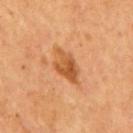<tbp_lesion>
  <patient>
    <sex>male</sex>
    <age_approx>55</age_approx>
  </patient>
  <site>back</site>
  <image>
    <source>total-body photography crop</source>
    <field_of_view_mm>15</field_of_view_mm>
  </image>
</tbp_lesion>